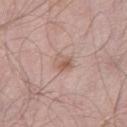| field | value |
|---|---|
| notes | catalogued during a skin exam; not biopsied |
| body site | the left thigh |
| imaging modality | 15 mm crop, total-body photography |
| subject | male, in their mid- to late 50s |